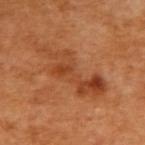follow-up: total-body-photography surveillance lesion; no biopsy | location: the upper back | patient: female, in their mid- to late 50s | image-analysis metrics: border irregularity of about 9 on a 0–10 scale, a within-lesion color-variation index near 7.5/10, and peripheral color asymmetry of about 2.5 | image: ~15 mm tile from a whole-body skin photo.